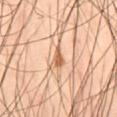The lesion was tiled from a total-body skin photograph and was not biopsied. The lesion is located on the right thigh. Cropped from a total-body skin-imaging series; the visible field is about 15 mm. A male patient, about 50 years old. This is a cross-polarized tile. About 2.5 mm across. The lesion-visualizer software estimated a mean CIELAB color near L≈62 a*≈22 b*≈37, a lesion–skin lightness drop of about 13, and a normalized lesion–skin contrast near 8.5. And it measured a detector confidence of about 100 out of 100 that the crop contains a lesion.